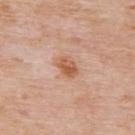Case summary:
* workup: imaged on a skin check; not biopsied
* body site: the upper back
* size: about 3 mm
* image source: ~15 mm crop, total-body skin-cancer survey
* patient: male, about 75 years old
* lighting: white-light